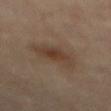| field | value |
|---|---|
| biopsy status | imaged on a skin check; not biopsied |
| patient | male, about 85 years old or older |
| acquisition | total-body-photography crop, ~15 mm field of view |
| lesion diameter | ≈5 mm |
| location | the mid back |
| lighting | cross-polarized |
| TBP lesion metrics | an area of roughly 12 mm², an eccentricity of roughly 0.75, and a symmetry-axis asymmetry near 0.3; an average lesion color of about L≈37 a*≈14 b*≈25 (CIELAB); a border-irregularity rating of about 3.5/10 and internal color variation of about 3.5 on a 0–10 scale |